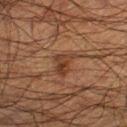| key | value |
|---|---|
| follow-up | no biopsy performed (imaged during a skin exam) |
| image-analysis metrics | an automated nevus-likeness rating near 5 out of 100 and lesion-presence confidence of about 100/100 |
| body site | the right lower leg |
| diameter | ~3.5 mm (longest diameter) |
| patient | male, aged 48–52 |
| image source | ~15 mm crop, total-body skin-cancer survey |
| illumination | cross-polarized |